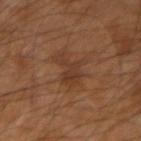This lesion was catalogued during total-body skin photography and was not selected for biopsy. The tile uses cross-polarized illumination. A male patient in their 60s. Located on the left forearm. A close-up tile cropped from a whole-body skin photograph, about 15 mm across. Approximately 4.5 mm at its widest.